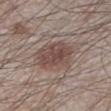– follow-up: total-body-photography surveillance lesion; no biopsy
– imaging modality: total-body-photography crop, ~15 mm field of view
– subject: male, in their 60s
– anatomic site: the leg
– lesion size: ≈5 mm
– tile lighting: white-light
– automated metrics: a lesion area of about 15 mm², an outline eccentricity of about 0.65 (0 = round, 1 = elongated), and a shape-asymmetry score of about 0.25 (0 = symmetric); a lesion color around L≈47 a*≈17 b*≈21 in CIELAB and a normalized lesion–skin contrast near 7.5